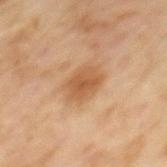biopsy status = imaged on a skin check; not biopsied
anatomic site = the upper back
subject = female, roughly 60 years of age
lesion size = about 4 mm
imaging modality = total-body-photography crop, ~15 mm field of view